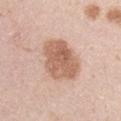<lesion>
  <biopsy_status>not biopsied; imaged during a skin examination</biopsy_status>
  <lighting>white-light</lighting>
  <patient>
    <sex>male</sex>
    <age_approx>45</age_approx>
  </patient>
  <image>
    <source>total-body photography crop</source>
    <field_of_view_mm>15</field_of_view_mm>
  </image>
  <site>right upper arm</site>
</lesion>